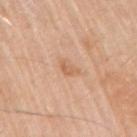Findings:
- biopsy status · total-body-photography surveillance lesion; no biopsy
- size · ~2.5 mm (longest diameter)
- body site · the right upper arm
- patient · male, approximately 80 years of age
- acquisition · ~15 mm crop, total-body skin-cancer survey
- TBP lesion metrics · an outline eccentricity of about 0.7 (0 = round, 1 = elongated); border irregularity of about 3 on a 0–10 scale and a color-variation rating of about 2/10
- lighting · white-light